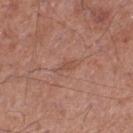Case summary:
- notes: catalogued during a skin exam; not biopsied
- patient: male, in their mid- to late 50s
- lesion size: ~2.5 mm (longest diameter)
- site: the left thigh
- acquisition: ~15 mm tile from a whole-body skin photo
- image-analysis metrics: an average lesion color of about L≈50 a*≈22 b*≈27 (CIELAB), a lesion–skin lightness drop of about 6, and a lesion-to-skin contrast of about 4.5 (normalized; higher = more distinct); a border-irregularity index near 3.5/10, a color-variation rating of about 0/10, and a peripheral color-asymmetry measure near 0